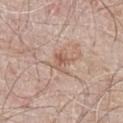Assessment: Captured during whole-body skin photography for melanoma surveillance; the lesion was not biopsied. Image and clinical context: The lesion is located on the chest. The tile uses white-light illumination. A roughly 15 mm field-of-view crop from a total-body skin photograph. Measured at roughly 2.5 mm in maximum diameter. A male patient aged approximately 65.